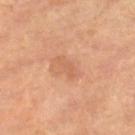Assessment: The lesion was photographed on a routine skin check and not biopsied; there is no pathology result. Background: A lesion tile, about 15 mm wide, cut from a 3D total-body photograph. On the left thigh. A female patient, in their 70s. Automated tile analysis of the lesion measured a border-irregularity rating of about 5.5/10, internal color variation of about 0 on a 0–10 scale, and a peripheral color-asymmetry measure near 0. It also reported a nevus-likeness score of about 0/100. Captured under cross-polarized illumination.